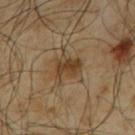The lesion was tiled from a total-body skin photograph and was not biopsied. From the upper back. Measured at roughly 3 mm in maximum diameter. The lesion-visualizer software estimated an area of roughly 6.5 mm², a shape eccentricity near 0.65, and two-axis asymmetry of about 0.3. The software also gave a lesion color around L≈37 a*≈15 b*≈30 in CIELAB, a lesion–skin lightness drop of about 9, and a normalized lesion–skin contrast near 8. A 15 mm close-up tile from a total-body photography series done for melanoma screening. A male subject in their mid-60s. Imaged with cross-polarized lighting.